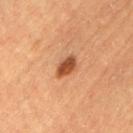Assessment:
This lesion was catalogued during total-body skin photography and was not selected for biopsy.
Context:
This is a cross-polarized tile. A female patient aged 58–62. The lesion is located on the left thigh. A 15 mm close-up tile from a total-body photography series done for melanoma screening. The lesion-visualizer software estimated an average lesion color of about L≈53 a*≈29 b*≈40 (CIELAB), about 16 CIELAB-L* units darker than the surrounding skin, and a normalized lesion–skin contrast near 10.5. The software also gave internal color variation of about 2.5 on a 0–10 scale.